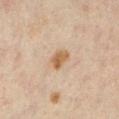Clinical impression:
Captured during whole-body skin photography for melanoma surveillance; the lesion was not biopsied.
Image and clinical context:
Approximately 2.5 mm at its widest. Imaged with cross-polarized lighting. An algorithmic analysis of the crop reported an area of roughly 4.5 mm², an outline eccentricity of about 0.7 (0 = round, 1 = elongated), and two-axis asymmetry of about 0.3. The analysis additionally found about 9 CIELAB-L* units darker than the surrounding skin and a normalized lesion–skin contrast near 8.5. It also reported border irregularity of about 2.5 on a 0–10 scale, internal color variation of about 3 on a 0–10 scale, and a peripheral color-asymmetry measure near 1. The software also gave a classifier nevus-likeness of about 90/100. A female subject aged approximately 45. A roughly 15 mm field-of-view crop from a total-body skin photograph. On the left lower leg.